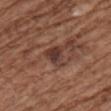This lesion was catalogued during total-body skin photography and was not selected for biopsy. Cropped from a total-body skin-imaging series; the visible field is about 15 mm. Approximately 3 mm at its widest. A female subject in their mid-70s. On the left upper arm.